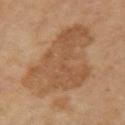Assessment: The lesion was photographed on a routine skin check and not biopsied; there is no pathology result. Clinical summary: The lesion is on the left arm. A female patient aged around 65. Cropped from a total-body skin-imaging series; the visible field is about 15 mm.